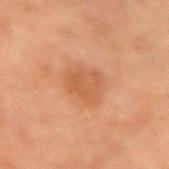The lesion was tiled from a total-body skin photograph and was not biopsied. The tile uses cross-polarized illumination. A 15 mm crop from a total-body photograph taken for skin-cancer surveillance. A male patient, roughly 60 years of age. Approximately 3.5 mm at its widest. The total-body-photography lesion software estimated an outline eccentricity of about 0.55 (0 = round, 1 = elongated). And it measured roughly 6 lightness units darker than nearby skin and a normalized border contrast of about 5.5. The lesion is on the left forearm.